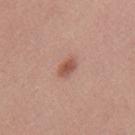workup: imaged on a skin check; not biopsied
site: the mid back
imaging modality: 15 mm crop, total-body photography
patient: female, aged 23–27
lesion diameter: about 2.5 mm